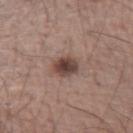body site — the arm | acquisition — ~15 mm crop, total-body skin-cancer survey | subject — male, in their mid-60s | size — ≈3 mm.